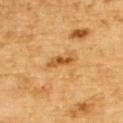site: back
patient:
  sex: male
  age_approx: 85+
image:
  source: total-body photography crop
  field_of_view_mm: 15
lighting: cross-polarized
lesion_size:
  long_diameter_mm_approx: 3.5
automated_metrics:
  area_mm2_approx: 4.5
  eccentricity: 0.9
  shape_asymmetry: 0.35
  border_irregularity_0_10: 4.0
  color_variation_0_10: 4.0
  peripheral_color_asymmetry: 1.0